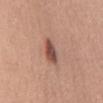The lesion was tiled from a total-body skin photograph and was not biopsied. The lesion is on the abdomen. The subject is a female aged 33–37. A region of skin cropped from a whole-body photographic capture, roughly 15 mm wide.